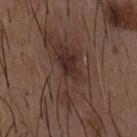Clinical impression: Part of a total-body skin-imaging series; this lesion was reviewed on a skin check and was not flagged for biopsy. Clinical summary: The recorded lesion diameter is about 9.5 mm. A 15 mm crop from a total-body photograph taken for skin-cancer surveillance. An algorithmic analysis of the crop reported an average lesion color of about L≈33 a*≈16 b*≈21 (CIELAB), a lesion–skin lightness drop of about 8, and a normalized lesion–skin contrast near 7.5. And it measured a classifier nevus-likeness of about 10/100 and a detector confidence of about 80 out of 100 that the crop contains a lesion. The tile uses white-light illumination. A male subject, aged 48 to 52. Located on the front of the torso.